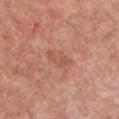Captured during whole-body skin photography for melanoma surveillance; the lesion was not biopsied.
Automated tile analysis of the lesion measured an area of roughly 4 mm², an eccentricity of roughly 0.85, and a symmetry-axis asymmetry near 0.4. And it measured border irregularity of about 4.5 on a 0–10 scale, a color-variation rating of about 2/10, and peripheral color asymmetry of about 0.5.
The patient is a male roughly 55 years of age.
About 3 mm across.
Captured under white-light illumination.
A region of skin cropped from a whole-body photographic capture, roughly 15 mm wide.
From the chest.